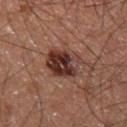<tbp_lesion>
<biopsy_status>not biopsied; imaged during a skin examination</biopsy_status>
<image>
  <source>total-body photography crop</source>
  <field_of_view_mm>15</field_of_view_mm>
</image>
<patient>
  <sex>male</sex>
  <age_approx>60</age_approx>
</patient>
<site>right thigh</site>
</tbp_lesion>